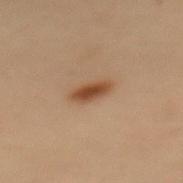Recorded during total-body skin imaging; not selected for excision or biopsy. A lesion tile, about 15 mm wide, cut from a 3D total-body photograph. The tile uses cross-polarized illumination. A female patient in their 50s. Located on the mid back. The recorded lesion diameter is about 3.5 mm.